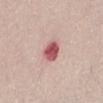Clinical impression: The lesion was tiled from a total-body skin photograph and was not biopsied. Context: Located on the front of the torso. Cropped from a total-body skin-imaging series; the visible field is about 15 mm. The patient is a male aged around 65.